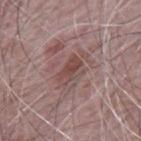<record>
<biopsy_status>not biopsied; imaged during a skin examination</biopsy_status>
<patient>
  <sex>male</sex>
  <age_approx>65</age_approx>
</patient>
<lesion_size>
  <long_diameter_mm_approx>3.5</long_diameter_mm_approx>
</lesion_size>
<lighting>white-light</lighting>
<image>
  <source>total-body photography crop</source>
  <field_of_view_mm>15</field_of_view_mm>
</image>
<site>mid back</site>
</record>